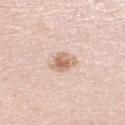<lesion>
  <lighting>white-light</lighting>
  <site>left lower leg</site>
  <image>
    <source>total-body photography crop</source>
    <field_of_view_mm>15</field_of_view_mm>
  </image>
  <patient>
    <sex>female</sex>
    <age_approx>45</age_approx>
  </patient>
</lesion>